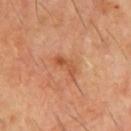Part of a total-body skin-imaging series; this lesion was reviewed on a skin check and was not flagged for biopsy. A close-up tile cropped from a whole-body skin photograph, about 15 mm across. An algorithmic analysis of the crop reported a mean CIELAB color near L≈49 a*≈26 b*≈36, a lesion–skin lightness drop of about 8, and a normalized lesion–skin contrast near 6.5. The analysis additionally found a border-irregularity rating of about 5.5/10 and a peripheral color-asymmetry measure near 0. The subject is a male aged around 60. Approximately 3 mm at its widest. From the chest.